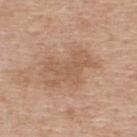This lesion was catalogued during total-body skin photography and was not selected for biopsy.
A male subject, aged 58–62.
A 15 mm close-up extracted from a 3D total-body photography capture.
The lesion is located on the upper back.